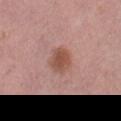Clinical impression: Imaged during a routine full-body skin examination; the lesion was not biopsied and no histopathology is available. Acquisition and patient details: A lesion tile, about 15 mm wide, cut from a 3D total-body photograph. A female patient about 40 years old. Automated tile analysis of the lesion measured an area of roughly 7.5 mm² and a shape eccentricity near 0.5. And it measured a border-irregularity index near 2/10, a within-lesion color-variation index near 3/10, and peripheral color asymmetry of about 1. On the right thigh. The recorded lesion diameter is about 3 mm. Imaged with white-light lighting.